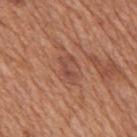Notes:
• workup: imaged on a skin check; not biopsied
• body site: the mid back
• patient: male, in their mid- to late 60s
• acquisition: ~15 mm tile from a whole-body skin photo